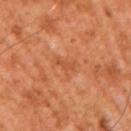lighting — cross-polarized | subject — male, approximately 65 years of age | image — ~15 mm crop, total-body skin-cancer survey | TBP lesion metrics — an outline eccentricity of about 0.95 (0 = round, 1 = elongated) and two-axis asymmetry of about 0.45; about 7 CIELAB-L* units darker than the surrounding skin and a normalized border contrast of about 5 | body site — the right upper arm.